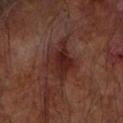| key | value |
|---|---|
| notes | total-body-photography surveillance lesion; no biopsy |
| image | 15 mm crop, total-body photography |
| subject | male, aged around 65 |
| anatomic site | the left forearm |